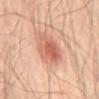{"biopsy_status": "not biopsied; imaged during a skin examination", "image": {"source": "total-body photography crop", "field_of_view_mm": 15}, "site": "front of the torso", "patient": {"sex": "male", "age_approx": 55}, "lesion_size": {"long_diameter_mm_approx": 4.5}, "lighting": "cross-polarized"}